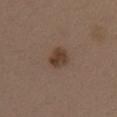Part of a total-body skin-imaging series; this lesion was reviewed on a skin check and was not flagged for biopsy.
An algorithmic analysis of the crop reported a shape eccentricity near 0.6 and two-axis asymmetry of about 0.25. And it measured about 10 CIELAB-L* units darker than the surrounding skin and a normalized lesion–skin contrast near 8.5.
This image is a 15 mm lesion crop taken from a total-body photograph.
The lesion is located on the arm.
A male subject roughly 40 years of age.